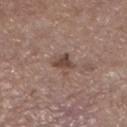Captured during whole-body skin photography for melanoma surveillance; the lesion was not biopsied. This image is a 15 mm lesion crop taken from a total-body photograph. The subject is a female aged 63 to 67. Imaged with white-light lighting. The lesion's longest dimension is about 2.5 mm. The lesion is located on the left lower leg.